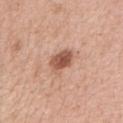<lesion>
<biopsy_status>not biopsied; imaged during a skin examination</biopsy_status>
<patient>
  <sex>female</sex>
  <age_approx>50</age_approx>
</patient>
<automated_metrics>
  <cielab_L>55</cielab_L>
  <cielab_a>23</cielab_a>
  <cielab_b>30</cielab_b>
  <vs_skin_contrast_norm>9.0</vs_skin_contrast_norm>
</automated_metrics>
<site>right upper arm</site>
<image>
  <source>total-body photography crop</source>
  <field_of_view_mm>15</field_of_view_mm>
</image>
</lesion>